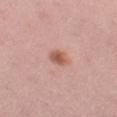  biopsy_status: not biopsied; imaged during a skin examination
  site: left thigh
  patient:
    sex: female
    age_approx: 35
  lesion_size:
    long_diameter_mm_approx: 2.5
  image:
    source: total-body photography crop
    field_of_view_mm: 15